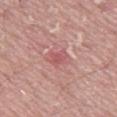Q: Was a biopsy performed?
A: total-body-photography surveillance lesion; no biopsy
Q: How was the tile lit?
A: white-light illumination
Q: What is the anatomic site?
A: the abdomen
Q: What kind of image is this?
A: 15 mm crop, total-body photography
Q: Lesion size?
A: about 2.5 mm
Q: Who is the patient?
A: male, roughly 40 years of age